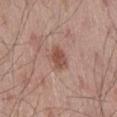Notes:
• workup · total-body-photography surveillance lesion; no biopsy
• patient · male, aged 53 to 57
• tile lighting · white-light
• body site · the right thigh
• diameter · ≈2.5 mm
• image-analysis metrics · an area of roughly 4 mm² and a symmetry-axis asymmetry near 0.2; a lesion-detection confidence of about 100/100
• image · 15 mm crop, total-body photography